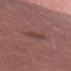notes: imaged on a skin check; not biopsied
TBP lesion metrics: a shape eccentricity near 0.75 and two-axis asymmetry of about 0.35; a border-irregularity rating of about 3.5/10, a within-lesion color-variation index near 1.5/10, and a peripheral color-asymmetry measure near 0.5; an automated nevus-likeness rating near 0 out of 100
site: the right thigh
image source: ~15 mm crop, total-body skin-cancer survey
subject: female, aged 58–62
diameter: ~3 mm (longest diameter)